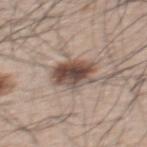{
  "biopsy_status": "not biopsied; imaged during a skin examination",
  "lighting": "white-light",
  "image": {
    "source": "total-body photography crop",
    "field_of_view_mm": 15
  },
  "automated_metrics": {
    "eccentricity": 0.7,
    "shape_asymmetry": 0.2,
    "cielab_L": 48,
    "cielab_a": 15,
    "cielab_b": 23,
    "vs_skin_darker_L": 16.0,
    "vs_skin_contrast_norm": 11.0,
    "border_irregularity_0_10": 2.5,
    "color_variation_0_10": 7.5
  },
  "lesion_size": {
    "long_diameter_mm_approx": 5.0
  },
  "site": "upper back",
  "patient": {
    "sex": "male",
    "age_approx": 65
  }
}